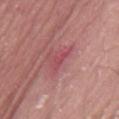Clinical impression:
The lesion was tiled from a total-body skin photograph and was not biopsied.
Acquisition and patient details:
The subject is a male in their 40s. Automated tile analysis of the lesion measured an eccentricity of roughly 0.7 and a symmetry-axis asymmetry near 0.5. The recorded lesion diameter is about 2 mm. The lesion is located on the back. A 15 mm close-up extracted from a 3D total-body photography capture. Captured under white-light illumination.